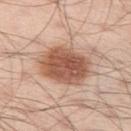workup = imaged on a skin check; not biopsied
anatomic site = the left thigh
acquisition = ~15 mm tile from a whole-body skin photo
tile lighting = white-light
subject = male, roughly 55 years of age
automated metrics = a footprint of about 22 mm² and two-axis asymmetry of about 0.15; a mean CIELAB color near L≈56 a*≈22 b*≈30 and roughly 15 lightness units darker than nearby skin; internal color variation of about 5.5 on a 0–10 scale; a nevus-likeness score of about 100/100 and lesion-presence confidence of about 100/100